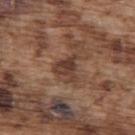<lesion>
  <biopsy_status>not biopsied; imaged during a skin examination</biopsy_status>
  <lesion_size>
    <long_diameter_mm_approx>3.0</long_diameter_mm_approx>
  </lesion_size>
  <site>upper back</site>
  <automated_metrics>
    <area_mm2_approx>5.5</area_mm2_approx>
    <eccentricity>0.3</eccentricity>
    <cielab_L>38</cielab_L>
    <cielab_a>19</cielab_a>
    <cielab_b>25</cielab_b>
    <vs_skin_darker_L>10.0</vs_skin_darker_L>
    <border_irregularity_0_10>5.0</border_irregularity_0_10>
    <color_variation_0_10>4.0</color_variation_0_10>
    <peripheral_color_asymmetry>1.0</peripheral_color_asymmetry>
  </automated_metrics>
  <image>
    <source>total-body photography crop</source>
    <field_of_view_mm>15</field_of_view_mm>
  </image>
  <patient>
    <sex>male</sex>
    <age_approx>75</age_approx>
  </patient>
  <lighting>white-light</lighting>
</lesion>